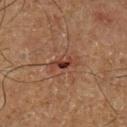Assessment: No biopsy was performed on this lesion — it was imaged during a full skin examination and was not determined to be concerning. Clinical summary: The lesion is located on the left lower leg. A close-up tile cropped from a whole-body skin photograph, about 15 mm across. Imaged with cross-polarized lighting. The lesion's longest dimension is about 3 mm. A male patient, aged approximately 65.